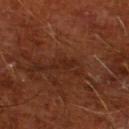Imaged during a routine full-body skin examination; the lesion was not biopsied and no histopathology is available.